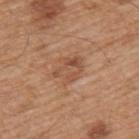Captured during whole-body skin photography for melanoma surveillance; the lesion was not biopsied.
Located on the back.
A male patient, roughly 65 years of age.
Cropped from a total-body skin-imaging series; the visible field is about 15 mm.